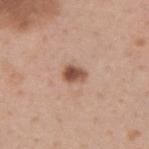The lesion was photographed on a routine skin check and not biopsied; there is no pathology result.
A male subject approximately 40 years of age.
A 15 mm close-up tile from a total-body photography series done for melanoma screening.
On the upper back.
The lesion-visualizer software estimated an area of roughly 4 mm² and an outline eccentricity of about 0.7 (0 = round, 1 = elongated). And it measured roughly 15 lightness units darker than nearby skin. The analysis additionally found a border-irregularity index near 2/10, a within-lesion color-variation index near 5/10, and a peripheral color-asymmetry measure near 2.
The tile uses white-light illumination.